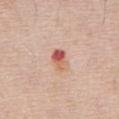workup = catalogued during a skin exam; not biopsied
diameter = ~3 mm (longest diameter)
illumination = white-light illumination
location = the abdomen
image source = total-body-photography crop, ~15 mm field of view
patient = male, aged around 70